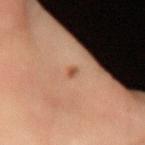Clinical impression:
The lesion was photographed on a routine skin check and not biopsied; there is no pathology result.
Background:
A female patient aged approximately 30. The tile uses cross-polarized illumination. Longest diameter approximately 1.5 mm. Located on the left forearm. Cropped from a whole-body photographic skin survey; the tile spans about 15 mm. Automated image analysis of the tile measured internal color variation of about 0 on a 0–10 scale and radial color variation of about 0. It also reported a nevus-likeness score of about 65/100 and lesion-presence confidence of about 100/100.